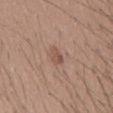biopsy status = catalogued during a skin exam; not biopsied
subject = male, aged approximately 40
site = the mid back
acquisition = 15 mm crop, total-body photography
lesion size = ~2.5 mm (longest diameter)
lighting = white-light illumination
TBP lesion metrics = a lesion area of about 3 mm², an eccentricity of roughly 0.85, and two-axis asymmetry of about 0.4; lesion-presence confidence of about 100/100